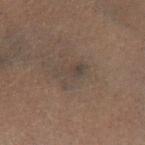No biopsy was performed on this lesion — it was imaged during a full skin examination and was not determined to be concerning.
Captured under cross-polarized illumination.
The lesion is on the left lower leg.
A male subject, aged 58–62.
The recorded lesion diameter is about 3.5 mm.
Cropped from a total-body skin-imaging series; the visible field is about 15 mm.